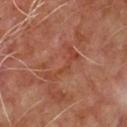{"biopsy_status": "not biopsied; imaged during a skin examination", "site": "chest", "image": {"source": "total-body photography crop", "field_of_view_mm": 15}, "patient": {"sex": "male", "age_approx": 65}}